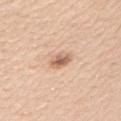follow-up: catalogued during a skin exam; not biopsied
size: ~3 mm (longest diameter)
tile lighting: white-light illumination
image source: total-body-photography crop, ~15 mm field of view
site: the left upper arm
patient: male, about 60 years old